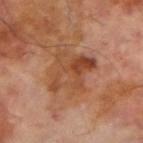workup: catalogued during a skin exam; not biopsied | illumination: cross-polarized illumination | patient: male, roughly 70 years of age | body site: the right upper arm | image: 15 mm crop, total-body photography.